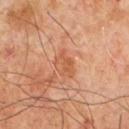The lesion was tiled from a total-body skin photograph and was not biopsied. This is a cross-polarized tile. This image is a 15 mm lesion crop taken from a total-body photograph. The lesion-visualizer software estimated border irregularity of about 3 on a 0–10 scale, a color-variation rating of about 3/10, and a peripheral color-asymmetry measure near 1. It also reported a nevus-likeness score of about 0/100 and a lesion-detection confidence of about 100/100. The recorded lesion diameter is about 3.5 mm. A male patient aged 63 to 67.